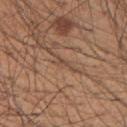* biopsy status: total-body-photography surveillance lesion; no biopsy
* image source: 15 mm crop, total-body photography
* lighting: white-light
* lesion size: ≈1.5 mm
* automated lesion analysis: a lesion area of about 1.5 mm² and a shape-asymmetry score of about 0.35 (0 = symmetric); an automated nevus-likeness rating near 0 out of 100
* subject: male, aged approximately 55
* body site: the right upper arm